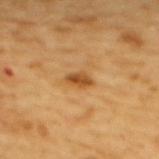Part of a total-body skin-imaging series; this lesion was reviewed on a skin check and was not flagged for biopsy. The tile uses cross-polarized illumination. A roughly 15 mm field-of-view crop from a total-body skin photograph. A female patient aged 53–57. On the upper back.